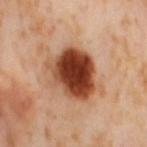Captured during whole-body skin photography for melanoma surveillance; the lesion was not biopsied. The lesion is on the right thigh. An algorithmic analysis of the crop reported a border-irregularity index near 2/10, internal color variation of about 9 on a 0–10 scale, and radial color variation of about 3. The patient is a female aged approximately 55. This is a cross-polarized tile. Measured at roughly 6 mm in maximum diameter. Cropped from a whole-body photographic skin survey; the tile spans about 15 mm.